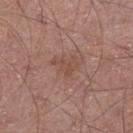This lesion was catalogued during total-body skin photography and was not selected for biopsy.
The lesion is on the right lower leg.
A male patient, aged around 70.
A 15 mm close-up tile from a total-body photography series done for melanoma screening.
Captured under white-light illumination.